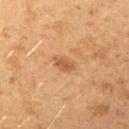Assessment:
Captured during whole-body skin photography for melanoma surveillance; the lesion was not biopsied.
Context:
Located on the left upper arm. Imaged with cross-polarized lighting. A female subject about 20 years old. A 15 mm close-up tile from a total-body photography series done for melanoma screening. About 2.5 mm across.